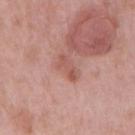workup: no biopsy performed (imaged during a skin exam); patient: male, roughly 55 years of age; location: the arm; image: 15 mm crop, total-body photography; lighting: white-light illumination.